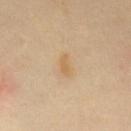Clinical impression:
Recorded during total-body skin imaging; not selected for excision or biopsy.
Image and clinical context:
A region of skin cropped from a whole-body photographic capture, roughly 15 mm wide. Imaged with cross-polarized lighting. On the chest. Measured at roughly 3 mm in maximum diameter. A female patient approximately 50 years of age.